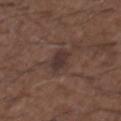{"biopsy_status": "not biopsied; imaged during a skin examination", "image": {"source": "total-body photography crop", "field_of_view_mm": 15}, "automated_metrics": {"border_irregularity_0_10": 3.0, "color_variation_0_10": 2.5, "peripheral_color_asymmetry": 1.0}, "lesion_size": {"long_diameter_mm_approx": 3.5}, "patient": {"sex": "male", "age_approx": 50}, "lighting": "white-light", "site": "back"}